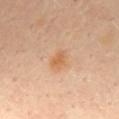workup = total-body-photography surveillance lesion; no biopsy | illumination = cross-polarized | site = the mid back | subject = female, about 60 years old | image = 15 mm crop, total-body photography | lesion diameter = about 2.5 mm.